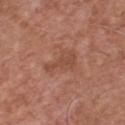{
  "biopsy_status": "not biopsied; imaged during a skin examination",
  "lesion_size": {
    "long_diameter_mm_approx": 4.0
  },
  "automated_metrics": {
    "area_mm2_approx": 4.5,
    "eccentricity": 0.95,
    "shape_asymmetry": 0.55,
    "nevus_likeness_0_100": 0,
    "lesion_detection_confidence_0_100": 100
  },
  "lighting": "white-light",
  "patient": {
    "sex": "male",
    "age_approx": 75
  },
  "image": {
    "source": "total-body photography crop",
    "field_of_view_mm": 15
  },
  "site": "chest"
}